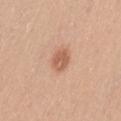Q: Is there a histopathology result?
A: total-body-photography surveillance lesion; no biopsy
Q: Automated lesion metrics?
A: an average lesion color of about L≈59 a*≈24 b*≈32 (CIELAB), roughly 11 lightness units darker than nearby skin, and a lesion-to-skin contrast of about 7 (normalized; higher = more distinct); border irregularity of about 1 on a 0–10 scale, internal color variation of about 3 on a 0–10 scale, and peripheral color asymmetry of about 1; a nevus-likeness score of about 95/100 and a lesion-detection confidence of about 100/100
Q: What is the lesion's diameter?
A: about 2.5 mm
Q: Where on the body is the lesion?
A: the lower back
Q: What kind of image is this?
A: ~15 mm crop, total-body skin-cancer survey
Q: Patient demographics?
A: male, about 30 years old